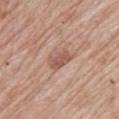Recorded during total-body skin imaging; not selected for excision or biopsy. The lesion is located on the upper back. Imaged with white-light lighting. A female subject, aged 68 to 72. Approximately 3 mm at its widest. A 15 mm close-up extracted from a 3D total-body photography capture.